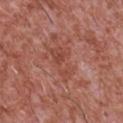{
  "biopsy_status": "not biopsied; imaged during a skin examination",
  "image": {
    "source": "total-body photography crop",
    "field_of_view_mm": 15
  },
  "patient": {
    "sex": "male",
    "age_approx": 45
  },
  "site": "chest",
  "lighting": "white-light",
  "lesion_size": {
    "long_diameter_mm_approx": 4.5
  },
  "automated_metrics": {
    "cielab_L": 47,
    "cielab_a": 26,
    "cielab_b": 29,
    "vs_skin_darker_L": 6.0,
    "nevus_likeness_0_100": 0,
    "lesion_detection_confidence_0_100": 100
  }
}